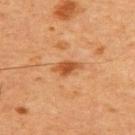biopsy_status: not biopsied; imaged during a skin examination
image:
  source: total-body photography crop
  field_of_view_mm: 15
patient:
  sex: male
  age_approx: 60
automated_metrics:
  area_mm2_approx: 4.0
  eccentricity: 0.85
  shape_asymmetry: 0.25
  color_variation_0_10: 2.0
  peripheral_color_asymmetry: 0.5
  nevus_likeness_0_100: 80
  lesion_detection_confidence_0_100: 100
lesion_size:
  long_diameter_mm_approx: 3.0
site: chest
lighting: cross-polarized